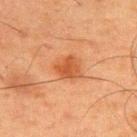Q: Is there a histopathology result?
A: imaged on a skin check; not biopsied
Q: Lesion location?
A: the back
Q: What kind of image is this?
A: ~15 mm tile from a whole-body skin photo
Q: Patient demographics?
A: male, in their 60s
Q: Automated lesion metrics?
A: an area of roughly 6.5 mm², an eccentricity of roughly 0.45, and a symmetry-axis asymmetry near 0.3; a border-irregularity rating of about 2.5/10, internal color variation of about 3 on a 0–10 scale, and radial color variation of about 1; a lesion-detection confidence of about 100/100
Q: Lesion size?
A: ~3 mm (longest diameter)